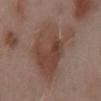Clinical impression: This lesion was catalogued during total-body skin photography and was not selected for biopsy. Context: Automated image analysis of the tile measured a lesion area of about 32 mm² and a symmetry-axis asymmetry near 0.4. It also reported a border-irregularity rating of about 5.5/10, a color-variation rating of about 5/10, and radial color variation of about 2. And it measured a classifier nevus-likeness of about 0/100. Cropped from a total-body skin-imaging series; the visible field is about 15 mm. Located on the back. The subject is a male about 55 years old. Approximately 9 mm at its widest.